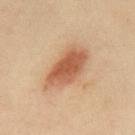Q: What are the patient's age and sex?
A: male, aged approximately 50
Q: What is the imaging modality?
A: 15 mm crop, total-body photography
Q: Where on the body is the lesion?
A: the mid back